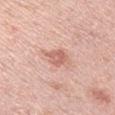Imaged during a routine full-body skin examination; the lesion was not biopsied and no histopathology is available.
The lesion is on the arm.
About 2.5 mm across.
The patient is a male approximately 45 years of age.
This image is a 15 mm lesion crop taken from a total-body photograph.
Imaged with white-light lighting.